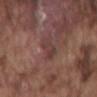- biopsy status — catalogued during a skin exam; not biopsied
- image source — ~15 mm tile from a whole-body skin photo
- body site — the mid back
- illumination — white-light illumination
- patient — male, aged 73 to 77
- size — ≈3.5 mm